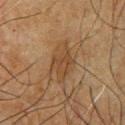Impression:
The lesion was photographed on a routine skin check and not biopsied; there is no pathology result.
Acquisition and patient details:
A 15 mm close-up tile from a total-body photography series done for melanoma screening. The tile uses cross-polarized illumination. From the chest. A male patient, approximately 65 years of age. The total-body-photography lesion software estimated a footprint of about 9.5 mm² and a shape-asymmetry score of about 0.25 (0 = symmetric). The analysis additionally found a border-irregularity rating of about 3.5/10, internal color variation of about 2 on a 0–10 scale, and peripheral color asymmetry of about 0.5. The analysis additionally found a classifier nevus-likeness of about 0/100 and lesion-presence confidence of about 95/100.